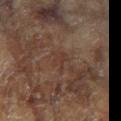The lesion was tiled from a total-body skin photograph and was not biopsied. A female patient aged 78 to 82. About 3 mm across. From the right arm. An algorithmic analysis of the crop reported a footprint of about 2.5 mm², a shape eccentricity near 0.85, and two-axis asymmetry of about 0.55. The analysis additionally found a lesion color around L≈32 a*≈17 b*≈22 in CIELAB, a lesion–skin lightness drop of about 4, and a normalized lesion–skin contrast near 4.5. The analysis additionally found border irregularity of about 6.5 on a 0–10 scale, a color-variation rating of about 0.5/10, and peripheral color asymmetry of about 0.5. The tile uses cross-polarized illumination. A 15 mm close-up extracted from a 3D total-body photography capture.